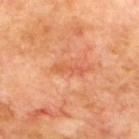Assessment: Part of a total-body skin-imaging series; this lesion was reviewed on a skin check and was not flagged for biopsy. Context: The lesion is located on the upper back. The patient is a male aged 68 to 72. Cropped from a whole-body photographic skin survey; the tile spans about 15 mm. The recorded lesion diameter is about 3.5 mm.